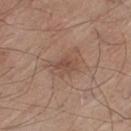notes — no biopsy performed (imaged during a skin exam) | TBP lesion metrics — an area of roughly 5.5 mm² and an outline eccentricity of about 0.75 (0 = round, 1 = elongated); a classifier nevus-likeness of about 10/100 and a detector confidence of about 100 out of 100 that the crop contains a lesion | tile lighting — white-light illumination | subject — male, about 80 years old | lesion size — ~3.5 mm (longest diameter) | site — the left thigh | imaging modality — ~15 mm tile from a whole-body skin photo.